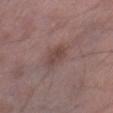Q: Was a biopsy performed?
A: imaged on a skin check; not biopsied
Q: What is the anatomic site?
A: the right thigh
Q: Lesion size?
A: about 3 mm
Q: What lighting was used for the tile?
A: white-light illumination
Q: Patient demographics?
A: male, about 50 years old
Q: What kind of image is this?
A: ~15 mm tile from a whole-body skin photo